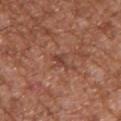This lesion was catalogued during total-body skin photography and was not selected for biopsy.
The lesion-visualizer software estimated a lesion color around L≈43 a*≈22 b*≈28 in CIELAB and a normalized border contrast of about 6.
Located on the front of the torso.
The tile uses white-light illumination.
Approximately 2.5 mm at its widest.
A region of skin cropped from a whole-body photographic capture, roughly 15 mm wide.
The subject is a male roughly 45 years of age.